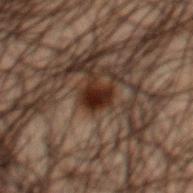Findings:
* notes: imaged on a skin check; not biopsied
* site: the mid back
* image: ~15 mm crop, total-body skin-cancer survey
* lighting: cross-polarized
* patient: male, aged 48 to 52
* lesion diameter: ≈2.5 mm